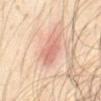Impression: The lesion was tiled from a total-body skin photograph and was not biopsied. Context: This is a cross-polarized tile. The total-body-photography lesion software estimated a footprint of about 9 mm² and a shape eccentricity near 0.8. And it measured a border-irregularity rating of about 3/10, internal color variation of about 3 on a 0–10 scale, and a peripheral color-asymmetry measure near 1. The analysis additionally found an automated nevus-likeness rating near 20 out of 100 and a detector confidence of about 100 out of 100 that the crop contains a lesion. Measured at roughly 4 mm in maximum diameter. A 15 mm close-up tile from a total-body photography series done for melanoma screening. A male patient aged approximately 70. The lesion is on the abdomen.